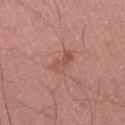No biopsy was performed on this lesion — it was imaged during a full skin examination and was not determined to be concerning.
On the left lower leg.
Automated tile analysis of the lesion measured an outline eccentricity of about 0.85 (0 = round, 1 = elongated) and a symmetry-axis asymmetry near 0.3. The software also gave an average lesion color of about L≈52 a*≈24 b*≈26 (CIELAB), about 7 CIELAB-L* units darker than the surrounding skin, and a lesion-to-skin contrast of about 5.5 (normalized; higher = more distinct). The software also gave a border-irregularity rating of about 3.5/10, a within-lesion color-variation index near 4/10, and peripheral color asymmetry of about 1.5. It also reported an automated nevus-likeness rating near 0 out of 100.
About 3 mm across.
A roughly 15 mm field-of-view crop from a total-body skin photograph.
A male subject aged 58–62.
The tile uses white-light illumination.